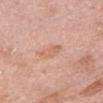Cropped from a whole-body photographic skin survey; the tile spans about 15 mm. About 3 mm across. The subject is a female aged approximately 60. Located on the head or neck. Imaged with white-light lighting.